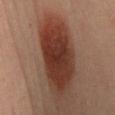Captured during whole-body skin photography for melanoma surveillance; the lesion was not biopsied.
Approximately 12 mm at its widest.
Located on the mid back.
The lesion-visualizer software estimated a lesion color around L≈36 a*≈22 b*≈27 in CIELAB, about 16 CIELAB-L* units darker than the surrounding skin, and a normalized lesion–skin contrast near 13. It also reported a detector confidence of about 100 out of 100 that the crop contains a lesion.
This image is a 15 mm lesion crop taken from a total-body photograph.
Imaged with cross-polarized lighting.
The patient is a female aged around 45.